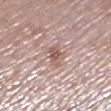Assessment: Imaged during a routine full-body skin examination; the lesion was not biopsied and no histopathology is available. Acquisition and patient details: A female subject, aged around 65. A lesion tile, about 15 mm wide, cut from a 3D total-body photograph. The total-body-photography lesion software estimated a lesion area of about 5 mm², an eccentricity of roughly 0.85, and a shape-asymmetry score of about 0.3 (0 = symmetric). The software also gave a normalized border contrast of about 7. The analysis additionally found a border-irregularity index near 3.5/10, a color-variation rating of about 3/10, and radial color variation of about 1. It also reported lesion-presence confidence of about 95/100. Measured at roughly 3.5 mm in maximum diameter. Located on the left lower leg. Imaged with white-light lighting.